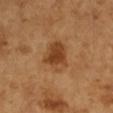Q: Was this lesion biopsied?
A: catalogued during a skin exam; not biopsied
Q: Lesion size?
A: ~4 mm (longest diameter)
Q: Where on the body is the lesion?
A: the arm
Q: What did automated image analysis measure?
A: an outline eccentricity of about 0.45 (0 = round, 1 = elongated); a detector confidence of about 100 out of 100 that the crop contains a lesion
Q: What lighting was used for the tile?
A: cross-polarized
Q: What is the imaging modality?
A: ~15 mm tile from a whole-body skin photo
Q: What are the patient's age and sex?
A: female, in their 70s